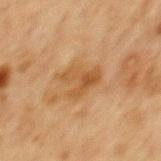Recorded during total-body skin imaging; not selected for excision or biopsy. Automated tile analysis of the lesion measured a footprint of about 9 mm², an eccentricity of roughly 0.65, and two-axis asymmetry of about 0.5. And it measured a lesion–skin lightness drop of about 8 and a normalized lesion–skin contrast near 6.5. It also reported border irregularity of about 6 on a 0–10 scale and peripheral color asymmetry of about 2. The analysis additionally found a detector confidence of about 100 out of 100 that the crop contains a lesion. Cropped from a whole-body photographic skin survey; the tile spans about 15 mm. The lesion is on the mid back. The subject is a male approximately 65 years of age. This is a cross-polarized tile.